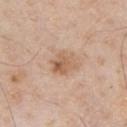* notes: total-body-photography surveillance lesion; no biopsy
* patient: male, aged around 40
* illumination: white-light illumination
* acquisition: ~15 mm tile from a whole-body skin photo
* anatomic site: the chest
* image-analysis metrics: a classifier nevus-likeness of about 30/100 and lesion-presence confidence of about 100/100